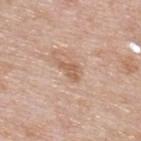Part of a total-body skin-imaging series; this lesion was reviewed on a skin check and was not flagged for biopsy.
Approximately 3 mm at its widest.
The patient is a male aged approximately 60.
The lesion is located on the upper back.
A 15 mm crop from a total-body photograph taken for skin-cancer surveillance.